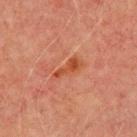workup=total-body-photography surveillance lesion; no biopsy
patient=male, aged around 70
imaging modality=~15 mm tile from a whole-body skin photo
diameter=~3.5 mm (longest diameter)
illumination=cross-polarized illumination
anatomic site=the front of the torso